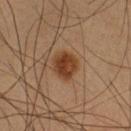Q: Lesion size?
A: ~3.5 mm (longest diameter)
Q: What are the patient's age and sex?
A: male, aged 58–62
Q: How was this image acquired?
A: total-body-photography crop, ~15 mm field of view
Q: Illumination type?
A: cross-polarized
Q: Automated lesion metrics?
A: a shape eccentricity near 0.6; a lesion color around L≈36 a*≈19 b*≈30 in CIELAB; border irregularity of about 1.5 on a 0–10 scale and a within-lesion color-variation index near 3.5/10; a nevus-likeness score of about 100/100 and a detector confidence of about 100 out of 100 that the crop contains a lesion
Q: Where on the body is the lesion?
A: the leg